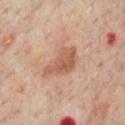The lesion was tiled from a total-body skin photograph and was not biopsied. A male subject, aged 63–67. The lesion is located on the front of the torso. Cropped from a total-body skin-imaging series; the visible field is about 15 mm.